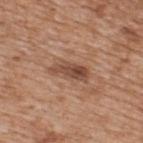Captured during whole-body skin photography for melanoma surveillance; the lesion was not biopsied.
Approximately 5 mm at its widest.
The lesion-visualizer software estimated an area of roughly 7.5 mm² and an eccentricity of roughly 0.9. The software also gave a border-irregularity rating of about 4/10, a within-lesion color-variation index near 6/10, and radial color variation of about 2.5. And it measured a classifier nevus-likeness of about 45/100 and lesion-presence confidence of about 100/100.
A region of skin cropped from a whole-body photographic capture, roughly 15 mm wide.
Captured under white-light illumination.
Located on the upper back.
The subject is a male aged 63–67.